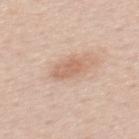Clinical impression:
Part of a total-body skin-imaging series; this lesion was reviewed on a skin check and was not flagged for biopsy.
Clinical summary:
A 15 mm close-up extracted from a 3D total-body photography capture. A female subject, aged around 40. On the mid back. An algorithmic analysis of the crop reported a footprint of about 6 mm², a shape eccentricity near 0.75, and a symmetry-axis asymmetry near 0.25. And it measured a border-irregularity rating of about 2.5/10, a color-variation rating of about 2.5/10, and a peripheral color-asymmetry measure near 1. It also reported a classifier nevus-likeness of about 35/100 and a lesion-detection confidence of about 100/100. Approximately 3 mm at its widest. The tile uses white-light illumination.